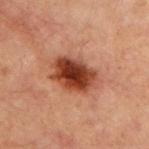The lesion was photographed on a routine skin check and not biopsied; there is no pathology result. The subject is a male aged around 70. Located on the chest. Cropped from a total-body skin-imaging series; the visible field is about 15 mm.